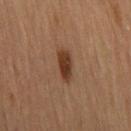Q: Is there a histopathology result?
A: imaged on a skin check; not biopsied
Q: What are the patient's age and sex?
A: female, aged 78–82
Q: What is the lesion's diameter?
A: about 4 mm
Q: Illumination type?
A: cross-polarized illumination
Q: Lesion location?
A: the right thigh
Q: How was this image acquired?
A: total-body-photography crop, ~15 mm field of view